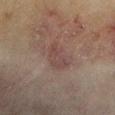Imaged with cross-polarized lighting.
A lesion tile, about 15 mm wide, cut from a 3D total-body photograph.
The lesion's longest dimension is about 4 mm.
From the left upper arm.
A male patient, in their 70s.
The total-body-photography lesion software estimated a border-irregularity index near 3.5/10, a color-variation rating of about 2.5/10, and peripheral color asymmetry of about 1.